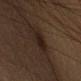Imaged during a routine full-body skin examination; the lesion was not biopsied and no histopathology is available.
A male subject, aged around 60.
The lesion is on the left upper arm.
Cropped from a whole-body photographic skin survey; the tile spans about 15 mm.